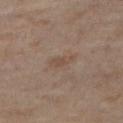* follow-up · catalogued during a skin exam; not biopsied
* subject · female, aged 48–52
* lesion size · about 3 mm
* TBP lesion metrics · a lesion area of about 3.5 mm², an outline eccentricity of about 0.9 (0 = round, 1 = elongated), and a symmetry-axis asymmetry near 0.3; border irregularity of about 3 on a 0–10 scale, internal color variation of about 1 on a 0–10 scale, and a peripheral color-asymmetry measure near 0; an automated nevus-likeness rating near 0 out of 100 and lesion-presence confidence of about 100/100
* location · the right thigh
* imaging modality · ~15 mm tile from a whole-body skin photo
* lighting · cross-polarized